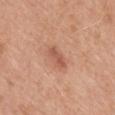  biopsy_status: not biopsied; imaged during a skin examination
  automated_metrics:
    cielab_L: 55
    cielab_a: 25
    cielab_b: 31
    vs_skin_darker_L: 9.0
    vs_skin_contrast_norm: 6.5
    border_irregularity_0_10: 3.0
    color_variation_0_10: 1.0
    peripheral_color_asymmetry: 0.0
  site: back
  patient:
    sex: male
    age_approx: 30
  image:
    source: total-body photography crop
    field_of_view_mm: 15